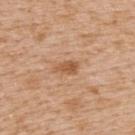Assessment: Captured during whole-body skin photography for melanoma surveillance; the lesion was not biopsied. Context: Automated tile analysis of the lesion measured a lesion color around L≈55 a*≈22 b*≈36 in CIELAB, roughly 10 lightness units darker than nearby skin, and a normalized border contrast of about 7.5. A lesion tile, about 15 mm wide, cut from a 3D total-body photograph. Located on the back. A male patient in their mid-60s. This is a white-light tile.